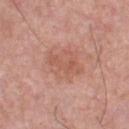Q: Was a biopsy performed?
A: no biopsy performed (imaged during a skin exam)
Q: How large is the lesion?
A: about 4 mm
Q: What is the anatomic site?
A: the front of the torso
Q: What is the imaging modality?
A: 15 mm crop, total-body photography
Q: How was the tile lit?
A: white-light
Q: Patient demographics?
A: male, aged approximately 45